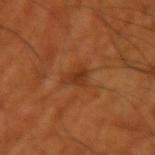Part of a total-body skin-imaging series; this lesion was reviewed on a skin check and was not flagged for biopsy.
A male patient, aged 68 to 72.
From the arm.
This image is a 15 mm lesion crop taken from a total-body photograph.